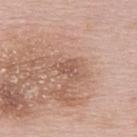Assessment: Imaged during a routine full-body skin examination; the lesion was not biopsied and no histopathology is available. Background: A 15 mm close-up extracted from a 3D total-body photography capture. Located on the upper back. The recorded lesion diameter is about 3 mm. A female subject, aged 63–67.